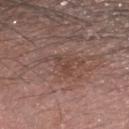| feature | finding |
|---|---|
| notes | catalogued during a skin exam; not biopsied |
| diameter | ~2.5 mm (longest diameter) |
| anatomic site | the head or neck |
| patient | male, in their mid-50s |
| illumination | white-light illumination |
| image source | ~15 mm crop, total-body skin-cancer survey |